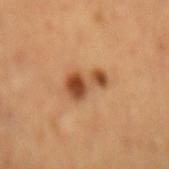The lesion was photographed on a routine skin check and not biopsied; there is no pathology result.
The recorded lesion diameter is about 4 mm.
This is a cross-polarized tile.
The lesion is located on the mid back.
An algorithmic analysis of the crop reported a lesion color around L≈49 a*≈24 b*≈37 in CIELAB, a lesion–skin lightness drop of about 14, and a normalized border contrast of about 10. It also reported a within-lesion color-variation index near 5/10.
A female subject.
A close-up tile cropped from a whole-body skin photograph, about 15 mm across.